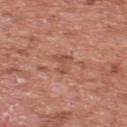  biopsy_status: not biopsied; imaged during a skin examination
  automated_metrics:
    area_mm2_approx: 4.0
    eccentricity: 0.8
    shape_asymmetry: 0.5
    cielab_L: 52
    cielab_a: 24
    cielab_b: 30
    vs_skin_darker_L: 7.0
    vs_skin_contrast_norm: 5.5
    border_irregularity_0_10: 5.0
    color_variation_0_10: 1.5
    peripheral_color_asymmetry: 0.5
    lesion_detection_confidence_0_100: 100
  patient:
    sex: male
    age_approx: 70
  site: back
  image:
    source: total-body photography crop
    field_of_view_mm: 15
  lighting: white-light